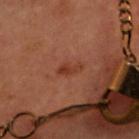Case summary:
* TBP lesion metrics: an area of roughly 3 mm², a shape eccentricity near 0.9, and a shape-asymmetry score of about 0.3 (0 = symmetric); a lesion–skin lightness drop of about 6; a border-irregularity rating of about 3.5/10, a color-variation rating of about 0.5/10, and peripheral color asymmetry of about 0
* acquisition: ~15 mm crop, total-body skin-cancer survey
* size: ~3 mm (longest diameter)
* subject: female, approximately 50 years of age
* location: the chest